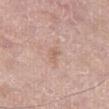<case>
  <biopsy_status>not biopsied; imaged during a skin examination</biopsy_status>
  <site>right thigh</site>
  <lesion_size>
    <long_diameter_mm_approx>2.5</long_diameter_mm_approx>
  </lesion_size>
  <patient>
    <sex>male</sex>
    <age_approx>80</age_approx>
  </patient>
  <image>
    <source>total-body photography crop</source>
    <field_of_view_mm>15</field_of_view_mm>
  </image>
  <lighting>white-light</lighting>
</case>